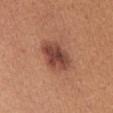Impression:
Part of a total-body skin-imaging series; this lesion was reviewed on a skin check and was not flagged for biopsy.
Background:
The total-body-photography lesion software estimated a lesion area of about 13 mm², an eccentricity of roughly 0.7, and a shape-asymmetry score of about 0.2 (0 = symmetric). And it measured border irregularity of about 2 on a 0–10 scale and radial color variation of about 1.5. A 15 mm close-up tile from a total-body photography series done for melanoma screening. Imaged with white-light lighting. The lesion is on the abdomen. Approximately 4.5 mm at its widest. The patient is a male aged 53–57.